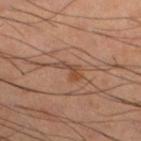notes=imaged on a skin check; not biopsied | size=about 3 mm | patient=male, aged around 40 | anatomic site=the left lower leg | lighting=cross-polarized | image source=~15 mm crop, total-body skin-cancer survey.